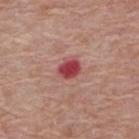Captured during whole-body skin photography for melanoma surveillance; the lesion was not biopsied. The tile uses white-light illumination. This image is a 15 mm lesion crop taken from a total-body photograph. The patient is a female aged 63–67. From the upper back. About 2.5 mm across.